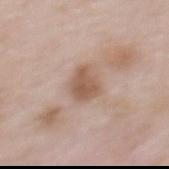Imaged during a routine full-body skin examination; the lesion was not biopsied and no histopathology is available. A female patient, about 65 years old. A close-up tile cropped from a whole-body skin photograph, about 15 mm across. Automated image analysis of the tile measured an area of roughly 7 mm² and a symmetry-axis asymmetry near 0.3. About 3.5 mm across. This is a white-light tile. Located on the upper back.